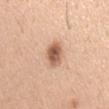A 15 mm crop from a total-body photograph taken for skin-cancer surveillance. A male patient, roughly 40 years of age. An algorithmic analysis of the crop reported an average lesion color of about L≈59 a*≈21 b*≈32 (CIELAB) and a lesion–skin lightness drop of about 15. It also reported a border-irregularity rating of about 2/10 and a within-lesion color-variation index near 5.5/10. And it measured a lesion-detection confidence of about 100/100. Longest diameter approximately 3.5 mm. This is a white-light tile. On the chest.